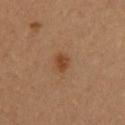Findings:
- workup — total-body-photography surveillance lesion; no biopsy
- automated lesion analysis — an average lesion color of about L≈41 a*≈21 b*≈33 (CIELAB), a lesion–skin lightness drop of about 9, and a normalized lesion–skin contrast near 8; a color-variation rating of about 2/10 and peripheral color asymmetry of about 0.5; a lesion-detection confidence of about 100/100
- lesion size — ~2.5 mm (longest diameter)
- lighting — cross-polarized
- subject — female, about 30 years old
- imaging modality — ~15 mm crop, total-body skin-cancer survey
- body site — the upper back